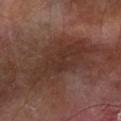Q: Was this lesion biopsied?
A: total-body-photography surveillance lesion; no biopsy
Q: Lesion location?
A: the left forearm
Q: What is the lesion's diameter?
A: ~9 mm (longest diameter)
Q: What kind of image is this?
A: ~15 mm crop, total-body skin-cancer survey
Q: Illumination type?
A: cross-polarized illumination
Q: What are the patient's age and sex?
A: male, aged 68 to 72